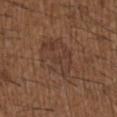The lesion was tiled from a total-body skin photograph and was not biopsied. The patient is a male aged 48 to 52. The lesion is located on the upper back. A roughly 15 mm field-of-view crop from a total-body skin photograph.